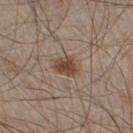Impression: Part of a total-body skin-imaging series; this lesion was reviewed on a skin check and was not flagged for biopsy.